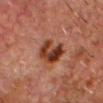The lesion was tiled from a total-body skin photograph and was not biopsied. Captured under cross-polarized illumination. A region of skin cropped from a whole-body photographic capture, roughly 15 mm wide. Measured at roughly 4.5 mm in maximum diameter. A male patient, about 65 years old. On the head or neck. The total-body-photography lesion software estimated a lesion color around L≈30 a*≈21 b*≈27 in CIELAB, a lesion–skin lightness drop of about 11, and a lesion-to-skin contrast of about 11 (normalized; higher = more distinct). And it measured a within-lesion color-variation index near 8.5/10. The analysis additionally found an automated nevus-likeness rating near 80 out of 100 and lesion-presence confidence of about 100/100.